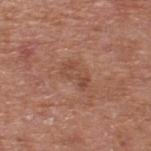No biopsy was performed on this lesion — it was imaged during a full skin examination and was not determined to be concerning. The lesion is located on the upper back. The lesion's longest dimension is about 3.5 mm. Automated tile analysis of the lesion measured an outline eccentricity of about 0.9 (0 = round, 1 = elongated). The software also gave a lesion color around L≈48 a*≈23 b*≈29 in CIELAB, about 7 CIELAB-L* units darker than the surrounding skin, and a lesion-to-skin contrast of about 5 (normalized; higher = more distinct). The analysis additionally found a border-irregularity index near 5/10, a within-lesion color-variation index near 2.5/10, and peripheral color asymmetry of about 0.5. The analysis additionally found a classifier nevus-likeness of about 0/100 and a detector confidence of about 100 out of 100 that the crop contains a lesion. A male patient aged 63–67. A 15 mm close-up tile from a total-body photography series done for melanoma screening. This is a white-light tile.